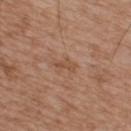Imaged during a routine full-body skin examination; the lesion was not biopsied and no histopathology is available. A male subject approximately 65 years of age. The lesion-visualizer software estimated a mean CIELAB color near L≈51 a*≈20 b*≈31, a lesion–skin lightness drop of about 6, and a normalized lesion–skin contrast near 5. The software also gave border irregularity of about 5 on a 0–10 scale, a color-variation rating of about 0.5/10, and peripheral color asymmetry of about 0. It also reported a nevus-likeness score of about 0/100 and a lesion-detection confidence of about 100/100. Captured under white-light illumination. From the upper back. Measured at roughly 3 mm in maximum diameter. A 15 mm close-up tile from a total-body photography series done for melanoma screening.